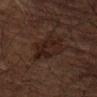Impression: Recorded during total-body skin imaging; not selected for excision or biopsy. Acquisition and patient details: The lesion is located on the back. This image is a 15 mm lesion crop taken from a total-body photograph. Captured under cross-polarized illumination. The recorded lesion diameter is about 4 mm. A male patient about 60 years old.